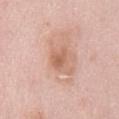Notes:
* notes — catalogued during a skin exam; not biopsied
* subject — female, in their 40s
* image — 15 mm crop, total-body photography
* lesion diameter — ~3 mm (longest diameter)
* illumination — white-light
* location — the mid back